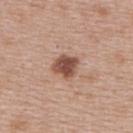notes = no biopsy performed (imaged during a skin exam); lesion size = ≈3 mm; illumination = white-light illumination; imaging modality = ~15 mm crop, total-body skin-cancer survey; site = the upper back; subject = female, aged 48–52.